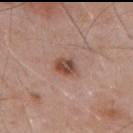workup — catalogued during a skin exam; not biopsied
location — the mid back
subject — male, aged 53 to 57
tile lighting — white-light illumination
image — 15 mm crop, total-body photography